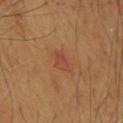Clinical impression: Part of a total-body skin-imaging series; this lesion was reviewed on a skin check and was not flagged for biopsy. Background: The lesion is located on the upper back. A male subject aged 63 to 67. Cropped from a whole-body photographic skin survey; the tile spans about 15 mm. The lesion's longest dimension is about 2.5 mm. Automated tile analysis of the lesion measured a shape eccentricity near 0.75 and two-axis asymmetry of about 0.35. It also reported a lesion color around L≈46 a*≈25 b*≈34 in CIELAB and a lesion–skin lightness drop of about 6. The analysis additionally found border irregularity of about 3 on a 0–10 scale, internal color variation of about 1.5 on a 0–10 scale, and a peripheral color-asymmetry measure near 0.5.